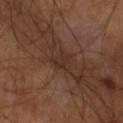biopsy status=total-body-photography surveillance lesion; no biopsy | acquisition=15 mm crop, total-body photography | illumination=cross-polarized | size=about 3 mm | body site=the left thigh | patient=male, roughly 60 years of age.